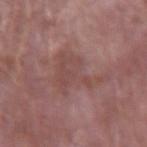No biopsy was performed on this lesion — it was imaged during a full skin examination and was not determined to be concerning. Automated tile analysis of the lesion measured a lesion color around L≈47 a*≈21 b*≈22 in CIELAB and a normalized border contrast of about 5. The analysis additionally found a border-irregularity rating of about 7.5/10 and radial color variation of about 0.5. It also reported a classifier nevus-likeness of about 0/100 and a detector confidence of about 80 out of 100 that the crop contains a lesion. A 15 mm close-up tile from a total-body photography series done for melanoma screening. The tile uses white-light illumination. A male subject, in their mid-60s. On the right forearm. Measured at roughly 7 mm in maximum diameter.